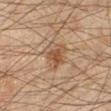Clinical impression:
Captured during whole-body skin photography for melanoma surveillance; the lesion was not biopsied.
Context:
A region of skin cropped from a whole-body photographic capture, roughly 15 mm wide. Automated tile analysis of the lesion measured a lesion-detection confidence of about 100/100. The tile uses cross-polarized illumination. Longest diameter approximately 3.5 mm. On the right lower leg. A male patient about 45 years old.